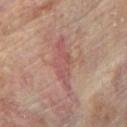The lesion-visualizer software estimated an average lesion color of about L≈51 a*≈23 b*≈23 (CIELAB), about 8 CIELAB-L* units darker than the surrounding skin, and a normalized lesion–skin contrast near 6. And it measured internal color variation of about 3 on a 0–10 scale. A male subject, aged 83 or over. On the upper back. A region of skin cropped from a whole-body photographic capture, roughly 15 mm wide. Measured at roughly 6.5 mm in maximum diameter.